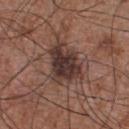The lesion was tiled from a total-body skin photograph and was not biopsied. From the chest. The tile uses white-light illumination. A lesion tile, about 15 mm wide, cut from a 3D total-body photograph. The lesion's longest dimension is about 5.5 mm. A male patient in their mid-50s.